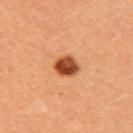• notes: no biopsy performed (imaged during a skin exam)
• body site: the right upper arm
• TBP lesion metrics: a mean CIELAB color near L≈47 a*≈30 b*≈40, a lesion–skin lightness drop of about 19, and a normalized lesion–skin contrast near 13; a within-lesion color-variation index near 5.5/10 and peripheral color asymmetry of about 2; a classifier nevus-likeness of about 100/100 and lesion-presence confidence of about 100/100
• lighting: cross-polarized illumination
• lesion size: about 2.5 mm
• subject: female, aged 33–37
• image: 15 mm crop, total-body photography